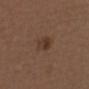• biopsy status — total-body-photography surveillance lesion; no biopsy
• size — ~3 mm (longest diameter)
• acquisition — total-body-photography crop, ~15 mm field of view
• patient — female, aged approximately 40
• site — the head or neck
• tile lighting — white-light illumination